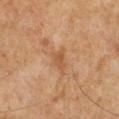Assessment: The lesion was photographed on a routine skin check and not biopsied; there is no pathology result. Acquisition and patient details: About 3 mm across. From the front of the torso. A close-up tile cropped from a whole-body skin photograph, about 15 mm across. The patient is a male aged 48 to 52.